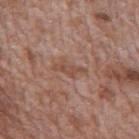Assessment:
No biopsy was performed on this lesion — it was imaged during a full skin examination and was not determined to be concerning.
Clinical summary:
Approximately 3.5 mm at its widest. The lesion is located on the mid back. A roughly 15 mm field-of-view crop from a total-body skin photograph. A male subject, in their 70s.